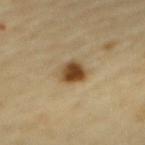Clinical impression: The lesion was photographed on a routine skin check and not biopsied; there is no pathology result.